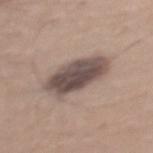No biopsy was performed on this lesion — it was imaged during a full skin examination and was not determined to be concerning.
On the mid back.
About 6.5 mm across.
A region of skin cropped from a whole-body photographic capture, roughly 15 mm wide.
Automated image analysis of the tile measured a lesion area of about 18 mm², a shape eccentricity near 0.85, and two-axis asymmetry of about 0.15. The software also gave a color-variation rating of about 4.5/10 and peripheral color asymmetry of about 1.5. The analysis additionally found a classifier nevus-likeness of about 30/100 and lesion-presence confidence of about 100/100.
The patient is a male approximately 30 years of age.